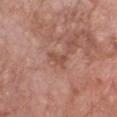Q: Patient demographics?
A: male, aged 78 to 82
Q: Where on the body is the lesion?
A: the chest
Q: Illumination type?
A: white-light illumination
Q: What is the lesion's diameter?
A: ≈2.5 mm
Q: How was this image acquired?
A: 15 mm crop, total-body photography